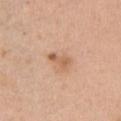Q: Was a biopsy performed?
A: no biopsy performed (imaged during a skin exam)
Q: Who is the patient?
A: female, aged approximately 40
Q: What is the anatomic site?
A: the chest
Q: What kind of image is this?
A: 15 mm crop, total-body photography